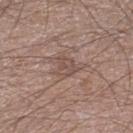A male subject, aged approximately 65.
A lesion tile, about 15 mm wide, cut from a 3D total-body photograph.
From the left lower leg.